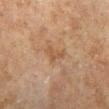The lesion was photographed on a routine skin check and not biopsied; there is no pathology result. The subject is a male roughly 65 years of age. Cropped from a whole-body photographic skin survey; the tile spans about 15 mm. The lesion is on the left lower leg.